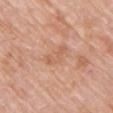Q: Was this lesion biopsied?
A: total-body-photography surveillance lesion; no biopsy